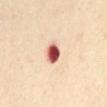Findings:
– follow-up: no biopsy performed (imaged during a skin exam)
– diameter: ≈2.5 mm
– lighting: cross-polarized illumination
– location: the abdomen
– image-analysis metrics: a lesion color around L≈59 a*≈32 b*≈29 in CIELAB, about 25 CIELAB-L* units darker than the surrounding skin, and a normalized border contrast of about 14.5; a border-irregularity index near 2/10, a color-variation rating of about 8/10, and peripheral color asymmetry of about 2
– patient: female, roughly 55 years of age
– imaging modality: 15 mm crop, total-body photography